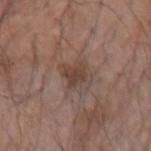follow-up — total-body-photography surveillance lesion; no biopsy
body site — the right upper arm
lesion size — ≈2.5 mm
tile lighting — white-light illumination
patient — male, aged 68–72
automated lesion analysis — a footprint of about 4.5 mm² and an eccentricity of roughly 0.45; an average lesion color of about L≈41 a*≈18 b*≈24 (CIELAB), roughly 9 lightness units darker than nearby skin, and a normalized border contrast of about 7
acquisition — ~15 mm crop, total-body skin-cancer survey